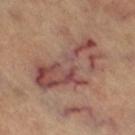No biopsy was performed on this lesion — it was imaged during a full skin examination and was not determined to be concerning. Automated tile analysis of the lesion measured a footprint of about 27 mm², an outline eccentricity of about 0.85 (0 = round, 1 = elongated), and two-axis asymmetry of about 0.4. The software also gave a border-irregularity rating of about 8/10, a color-variation rating of about 8/10, and peripheral color asymmetry of about 2.5. This is a cross-polarized tile. A female subject in their 60s. Approximately 8.5 mm at its widest. The lesion is on the left leg. A roughly 15 mm field-of-view crop from a total-body skin photograph.